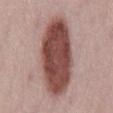tile lighting — white-light | patient — female, aged around 50 | TBP lesion metrics — a lesion area of about 40 mm², an outline eccentricity of about 0.85 (0 = round, 1 = elongated), and a shape-asymmetry score of about 0.1 (0 = symmetric); a mean CIELAB color near L≈47 a*≈22 b*≈23, a lesion–skin lightness drop of about 19, and a normalized lesion–skin contrast near 12.5; border irregularity of about 1.5 on a 0–10 scale, a within-lesion color-variation index near 5.5/10, and peripheral color asymmetry of about 2 | image — total-body-photography crop, ~15 mm field of view | lesion size — ≈9.5 mm | site — the mid back.